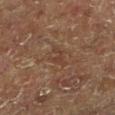Recorded during total-body skin imaging; not selected for excision or biopsy. Imaged with cross-polarized lighting. The recorded lesion diameter is about 3.5 mm. A roughly 15 mm field-of-view crop from a total-body skin photograph. The patient is a male in their mid-70s. An algorithmic analysis of the crop reported an average lesion color of about L≈31 a*≈15 b*≈22 (CIELAB) and roughly 4 lightness units darker than nearby skin. The analysis additionally found border irregularity of about 4 on a 0–10 scale, a color-variation rating of about 3/10, and peripheral color asymmetry of about 1. The software also gave a classifier nevus-likeness of about 0/100 and a lesion-detection confidence of about 80/100. From the left lower leg.